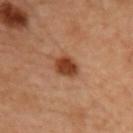notes: imaged on a skin check; not biopsied | image source: ~15 mm crop, total-body skin-cancer survey | anatomic site: the chest | lesion diameter: about 3.5 mm | automated lesion analysis: a border-irregularity index near 2/10, internal color variation of about 3 on a 0–10 scale, and radial color variation of about 1; lesion-presence confidence of about 100/100 | patient: female, aged 58–62.